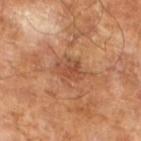Acquisition and patient details:
Imaged with cross-polarized lighting. The lesion-visualizer software estimated a lesion area of about 9 mm², an outline eccentricity of about 0.6 (0 = round, 1 = elongated), and a shape-asymmetry score of about 0.25 (0 = symmetric). The analysis additionally found a border-irregularity index near 3.5/10, internal color variation of about 4.5 on a 0–10 scale, and a peripheral color-asymmetry measure near 1.5. It also reported a classifier nevus-likeness of about 0/100 and a detector confidence of about 100 out of 100 that the crop contains a lesion. About 4 mm across. This image is a 15 mm lesion crop taken from a total-body photograph. A male subject, aged approximately 65.